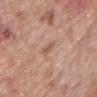{
  "biopsy_status": "not biopsied; imaged during a skin examination",
  "lesion_size": {
    "long_diameter_mm_approx": 3.0
  },
  "lighting": "white-light",
  "site": "chest",
  "patient": {
    "sex": "male",
    "age_approx": 60
  },
  "image": {
    "source": "total-body photography crop",
    "field_of_view_mm": 15
  },
  "automated_metrics": {
    "area_mm2_approx": 2.5,
    "eccentricity": 0.95,
    "shape_asymmetry": 0.45,
    "border_irregularity_0_10": 4.5,
    "color_variation_0_10": 0.0,
    "peripheral_color_asymmetry": 0.0,
    "nevus_likeness_0_100": 0,
    "lesion_detection_confidence_0_100": 95
  }
}